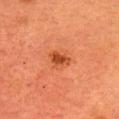Q: What did automated image analysis measure?
A: a border-irregularity rating of about 3.5/10, a color-variation rating of about 1.5/10, and a peripheral color-asymmetry measure near 0.5; a detector confidence of about 100 out of 100 that the crop contains a lesion
Q: Illumination type?
A: cross-polarized
Q: What is the lesion's diameter?
A: ≈3 mm
Q: Lesion location?
A: the head or neck
Q: Patient demographics?
A: female, in their mid-50s
Q: What kind of image is this?
A: total-body-photography crop, ~15 mm field of view